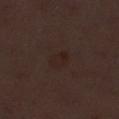A close-up tile cropped from a whole-body skin photograph, about 15 mm across. The tile uses white-light illumination. Longest diameter approximately 2.5 mm. Located on the left lower leg. The subject is a female approximately 50 years of age.